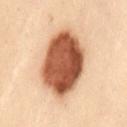biopsy status: imaged on a skin check; not biopsied | body site: the lower back | automated metrics: a footprint of about 33 mm² and an outline eccentricity of about 0.55 (0 = round, 1 = elongated); a lesion color around L≈43 a*≈21 b*≈29 in CIELAB, a lesion–skin lightness drop of about 20, and a lesion-to-skin contrast of about 14.5 (normalized; higher = more distinct) | tile lighting: cross-polarized illumination | lesion size: ~7 mm (longest diameter) | subject: female, aged around 30 | imaging modality: ~15 mm tile from a whole-body skin photo.